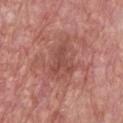The lesion was photographed on a routine skin check and not biopsied; there is no pathology result.
Cropped from a total-body skin-imaging series; the visible field is about 15 mm.
A male patient, aged around 65.
On the chest.
Measured at roughly 3.5 mm in maximum diameter.